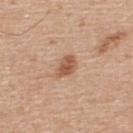Assessment: The lesion was photographed on a routine skin check and not biopsied; there is no pathology result. Clinical summary: The lesion is on the upper back. Automated image analysis of the tile measured a footprint of about 4.5 mm², an outline eccentricity of about 0.8 (0 = round, 1 = elongated), and two-axis asymmetry of about 0.25. It also reported an average lesion color of about L≈56 a*≈22 b*≈32 (CIELAB). The software also gave a border-irregularity rating of about 2/10 and a within-lesion color-variation index near 2.5/10. And it measured an automated nevus-likeness rating near 90 out of 100 and a detector confidence of about 100 out of 100 that the crop contains a lesion. A male subject, aged around 55. Cropped from a whole-body photographic skin survey; the tile spans about 15 mm. About 3 mm across. This is a white-light tile.